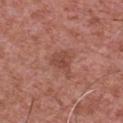Q: Is there a histopathology result?
A: total-body-photography surveillance lesion; no biopsy
Q: Automated lesion metrics?
A: a lesion color around L≈46 a*≈25 b*≈27 in CIELAB and a normalized border contrast of about 6; a classifier nevus-likeness of about 20/100
Q: Where on the body is the lesion?
A: the chest
Q: Illumination type?
A: white-light
Q: Lesion size?
A: about 3 mm
Q: What are the patient's age and sex?
A: male, roughly 45 years of age
Q: How was this image acquired?
A: total-body-photography crop, ~15 mm field of view